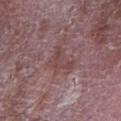This lesion was catalogued during total-body skin photography and was not selected for biopsy. Longest diameter approximately 4 mm. A male subject in their mid-60s. A 15 mm close-up tile from a total-body photography series done for melanoma screening. The lesion is located on the right forearm. This is a white-light tile.